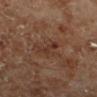Background: The total-body-photography lesion software estimated a lesion area of about 3.5 mm² and two-axis asymmetry of about 0.4. And it measured an average lesion color of about L≈32 a*≈20 b*≈25 (CIELAB), roughly 6 lightness units darker than nearby skin, and a lesion-to-skin contrast of about 6 (normalized; higher = more distinct). And it measured a within-lesion color-variation index near 0.5/10 and radial color variation of about 0. The software also gave a nevus-likeness score of about 0/100 and a lesion-detection confidence of about 100/100. A male patient, roughly 70 years of age. A close-up tile cropped from a whole-body skin photograph, about 15 mm across. Approximately 3.5 mm at its widest. Located on the leg. Captured under cross-polarized illumination.